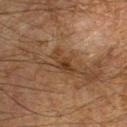image:
  source: total-body photography crop
  field_of_view_mm: 15
site: left forearm
patient:
  sex: male
  age_approx: 60
automated_metrics:
  area_mm2_approx: 4.5
  shape_asymmetry: 0.35
  color_variation_0_10: 2.5
  peripheral_color_asymmetry: 0.5
lesion_size:
  long_diameter_mm_approx: 3.5